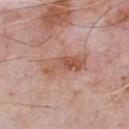follow-up: imaged on a skin check; not biopsied | image source: ~15 mm crop, total-body skin-cancer survey | automated metrics: an area of roughly 11 mm² and a symmetry-axis asymmetry near 0.25; a classifier nevus-likeness of about 0/100 and a lesion-detection confidence of about 100/100 | patient: male, roughly 60 years of age | site: the chest | lesion diameter: ≈5.5 mm.